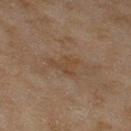Q: Is there a histopathology result?
A: catalogued during a skin exam; not biopsied
Q: Patient demographics?
A: female, roughly 60 years of age
Q: What kind of image is this?
A: ~15 mm crop, total-body skin-cancer survey
Q: What is the anatomic site?
A: the right thigh
Q: Automated lesion metrics?
A: border irregularity of about 5.5 on a 0–10 scale, a within-lesion color-variation index near 1.5/10, and a peripheral color-asymmetry measure near 0.5
Q: Lesion size?
A: ~3.5 mm (longest diameter)
Q: Illumination type?
A: cross-polarized illumination